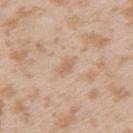workup: imaged on a skin check; not biopsied
image-analysis metrics: a lesion area of about 3 mm², a shape eccentricity near 0.85, and two-axis asymmetry of about 0.35; an average lesion color of about L≈63 a*≈17 b*≈33 (CIELAB) and a lesion–skin lightness drop of about 7; a nevus-likeness score of about 0/100 and a detector confidence of about 100 out of 100 that the crop contains a lesion
subject: female, in their mid-20s
anatomic site: the arm
imaging modality: ~15 mm tile from a whole-body skin photo
lighting: white-light
lesion diameter: about 2.5 mm